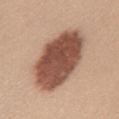  biopsy_status: not biopsied; imaged during a skin examination
  patient:
    sex: female
    age_approx: 35
  image:
    source: total-body photography crop
    field_of_view_mm: 15
  site: mid back